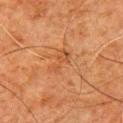{"biopsy_status": "not biopsied; imaged during a skin examination", "site": "chest", "patient": {"sex": "male", "age_approx": 80}, "image": {"source": "total-body photography crop", "field_of_view_mm": 15}, "lighting": "cross-polarized"}